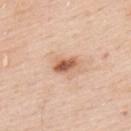{"biopsy_status": "not biopsied; imaged during a skin examination"}